This is a white-light tile. A male patient aged around 80. A roughly 15 mm field-of-view crop from a total-body skin photograph. Automated image analysis of the tile measured a classifier nevus-likeness of about 0/100. From the upper back.
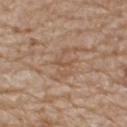histopathology: a nodular basal cell carcinoma — a malignancy.